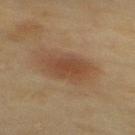This lesion was catalogued during total-body skin photography and was not selected for biopsy. A region of skin cropped from a whole-body photographic capture, roughly 15 mm wide. The lesion is on the back. This is a cross-polarized tile. The recorded lesion diameter is about 5 mm. The subject is a female aged 58–62.